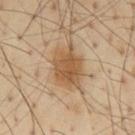workup: catalogued during a skin exam; not biopsied | image source: ~15 mm tile from a whole-body skin photo | subject: male, in their mid- to late 50s | site: the back | tile lighting: cross-polarized.